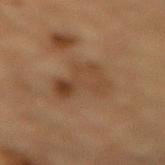Findings:
* workup · imaged on a skin check; not biopsied
* site · the lower back
* diameter · ~5 mm (longest diameter)
* patient · male, in their mid-80s or older
* lighting · cross-polarized
* imaging modality · 15 mm crop, total-body photography
* automated lesion analysis · an area of roughly 15 mm², an eccentricity of roughly 0.55, and a symmetry-axis asymmetry near 0.45; a lesion color around L≈37 a*≈16 b*≈28 in CIELAB and a lesion-to-skin contrast of about 5.5 (normalized; higher = more distinct); a border-irregularity index near 5/10, a color-variation rating of about 5/10, and a peripheral color-asymmetry measure near 2